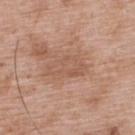Clinical impression: Recorded during total-body skin imaging; not selected for excision or biopsy. Acquisition and patient details: A 15 mm close-up extracted from a 3D total-body photography capture. An algorithmic analysis of the crop reported an eccentricity of roughly 0.9 and a shape-asymmetry score of about 0.35 (0 = symmetric). It also reported a border-irregularity index near 4/10. And it measured a nevus-likeness score of about 0/100. The lesion's longest dimension is about 5 mm. A male patient, about 50 years old. From the upper back.